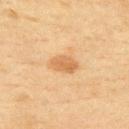Acquisition and patient details:
A female subject, approximately 60 years of age. From the back. Cropped from a whole-body photographic skin survey; the tile spans about 15 mm.